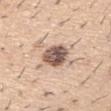biopsy_status: not biopsied; imaged during a skin examination
lesion_size:
  long_diameter_mm_approx: 3.5
image:
  source: total-body photography crop
  field_of_view_mm: 15
site: arm
patient:
  sex: male
  age_approx: 60